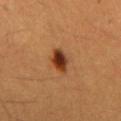This image is a 15 mm lesion crop taken from a total-body photograph. The lesion-visualizer software estimated a color-variation rating of about 6/10 and radial color variation of about 1.5. The analysis additionally found a classifier nevus-likeness of about 100/100 and lesion-presence confidence of about 100/100. The lesion is located on the mid back. The lesion's longest dimension is about 3.5 mm. Captured under cross-polarized illumination. A male subject roughly 60 years of age.